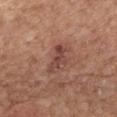notes = imaged on a skin check; not biopsied